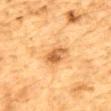Image and clinical context: A close-up tile cropped from a whole-body skin photograph, about 15 mm across. The tile uses cross-polarized illumination. The lesion is on the mid back. Longest diameter approximately 3.5 mm. A male subject aged approximately 85 or older.